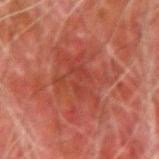Part of a total-body skin-imaging series; this lesion was reviewed on a skin check and was not flagged for biopsy.
A lesion tile, about 15 mm wide, cut from a 3D total-body photograph.
A male patient, aged approximately 80.
On the left forearm.
Measured at roughly 8 mm in maximum diameter.